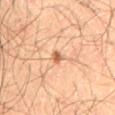Impression: Captured during whole-body skin photography for melanoma surveillance; the lesion was not biopsied. Context: This image is a 15 mm lesion crop taken from a total-body photograph. On the mid back. A male patient aged 53–57.